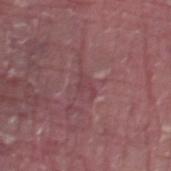{"biopsy_status": "not biopsied; imaged during a skin examination", "image": {"source": "total-body photography crop", "field_of_view_mm": 15}, "patient": {"sex": "male", "age_approx": 40}, "lesion_size": {"long_diameter_mm_approx": 2.5}, "site": "right thigh", "lighting": "white-light"}